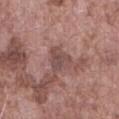lesion diameter: ~3.5 mm (longest diameter)
subject: male, roughly 75 years of age
tile lighting: white-light illumination
automated lesion analysis: an area of roughly 6.5 mm² and an outline eccentricity of about 0.55 (0 = round, 1 = elongated); a border-irregularity index near 4.5/10, internal color variation of about 2.5 on a 0–10 scale, and a peripheral color-asymmetry measure near 1; a nevus-likeness score of about 0/100
image source: 15 mm crop, total-body photography
site: the abdomen